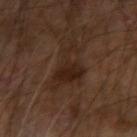Part of a total-body skin-imaging series; this lesion was reviewed on a skin check and was not flagged for biopsy. The lesion is located on the right arm. A 15 mm close-up extracted from a 3D total-body photography capture. Imaged with cross-polarized lighting. The patient is a male roughly 60 years of age. Approximately 7 mm at its widest. Automated tile analysis of the lesion measured a footprint of about 12 mm², a shape eccentricity near 0.9, and a symmetry-axis asymmetry near 0.6. And it measured a border-irregularity rating of about 8.5/10, internal color variation of about 2.5 on a 0–10 scale, and a peripheral color-asymmetry measure near 0.5.